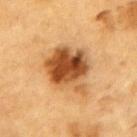Q: Was this lesion biopsied?
A: imaged on a skin check; not biopsied
Q: Where on the body is the lesion?
A: the upper back
Q: Who is the patient?
A: male, in their mid- to late 80s or older
Q: What is the imaging modality?
A: 15 mm crop, total-body photography